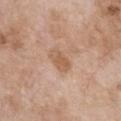| key | value |
|---|---|
| follow-up | imaged on a skin check; not biopsied |
| acquisition | ~15 mm crop, total-body skin-cancer survey |
| patient | female, aged around 75 |
| lesion size | ≈3 mm |
| tile lighting | white-light illumination |
| anatomic site | the chest |